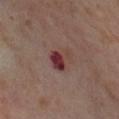Assessment:
This lesion was catalogued during total-body skin photography and was not selected for biopsy.
Background:
This is a cross-polarized tile. The recorded lesion diameter is about 3.5 mm. A female patient, in their mid-50s. Cropped from a whole-body photographic skin survey; the tile spans about 15 mm. The total-body-photography lesion software estimated roughly 12 lightness units darker than nearby skin. The software also gave a border-irregularity rating of about 4/10, internal color variation of about 5 on a 0–10 scale, and peripheral color asymmetry of about 1. It also reported a lesion-detection confidence of about 100/100. The lesion is located on the right thigh.